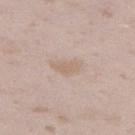{
  "biopsy_status": "not biopsied; imaged during a skin examination",
  "lesion_size": {
    "long_diameter_mm_approx": 2.5
  },
  "image": {
    "source": "total-body photography crop",
    "field_of_view_mm": 15
  },
  "automated_metrics": {
    "area_mm2_approx": 3.0,
    "eccentricity": 0.85,
    "shape_asymmetry": 0.4,
    "cielab_L": 63,
    "cielab_a": 15,
    "cielab_b": 27,
    "vs_skin_darker_L": 7.0,
    "vs_skin_contrast_norm": 5.5,
    "border_irregularity_0_10": 3.5,
    "color_variation_0_10": 0.5,
    "peripheral_color_asymmetry": 0.0
  },
  "site": "left thigh",
  "patient": {
    "sex": "female",
    "age_approx": 25
  }
}